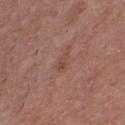A male patient in their mid-20s. A close-up tile cropped from a whole-body skin photograph, about 15 mm across. About 2.5 mm across. The lesion is located on the chest. The tile uses white-light illumination. The lesion-visualizer software estimated an automated nevus-likeness rating near 0 out of 100 and a detector confidence of about 100 out of 100 that the crop contains a lesion.